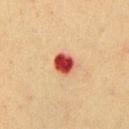notes: total-body-photography surveillance lesion; no biopsy
patient: male, about 55 years old
size: about 2.5 mm
site: the chest
image source: ~15 mm crop, total-body skin-cancer survey
TBP lesion metrics: an outline eccentricity of about 0.4 (0 = round, 1 = elongated); a border-irregularity rating of about 1/10, internal color variation of about 4 on a 0–10 scale, and radial color variation of about 1; a nevus-likeness score of about 0/100 and a detector confidence of about 100 out of 100 that the crop contains a lesion
lighting: cross-polarized illumination